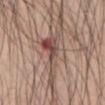– biopsy status · no biopsy performed (imaged during a skin exam)
– size · ~7.5 mm (longest diameter)
– patient · male, in their mid-60s
– image · ~15 mm crop, total-body skin-cancer survey
– location · the chest
– lighting · white-light